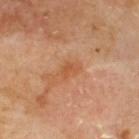<case>
  <biopsy_status>not biopsied; imaged during a skin examination</biopsy_status>
  <image>
    <source>total-body photography crop</source>
    <field_of_view_mm>15</field_of_view_mm>
  </image>
  <site>upper back</site>
  <lesion_size>
    <long_diameter_mm_approx>2.5</long_diameter_mm_approx>
  </lesion_size>
  <lighting>cross-polarized</lighting>
  <automated_metrics>
    <border_irregularity_0_10>4.0</border_irregularity_0_10>
    <color_variation_0_10>1.5</color_variation_0_10>
  </automated_metrics>
  <patient>
    <sex>male</sex>
    <age_approx>70</age_approx>
  </patient>
</case>